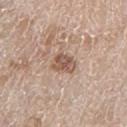The lesion was photographed on a routine skin check and not biopsied; there is no pathology result. The patient is a male roughly 80 years of age. Captured under white-light illumination. The lesion is on the left thigh. Longest diameter approximately 3 mm. A 15 mm close-up tile from a total-body photography series done for melanoma screening.